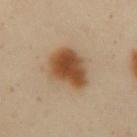The lesion was photographed on a routine skin check and not biopsied; there is no pathology result.
The lesion-visualizer software estimated a footprint of about 16 mm² and a shape eccentricity near 0.65. And it measured about 12 CIELAB-L* units darker than the surrounding skin and a normalized border contrast of about 10.5. The analysis additionally found an automated nevus-likeness rating near 100 out of 100.
Captured under cross-polarized illumination.
A lesion tile, about 15 mm wide, cut from a 3D total-body photograph.
From the abdomen.
A female patient, aged around 50.